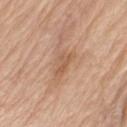Notes:
- lesion size · ≈2.5 mm
- tile lighting · white-light
- subject · male, aged approximately 70
- anatomic site · the left upper arm
- imaging modality · total-body-photography crop, ~15 mm field of view